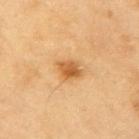Impression: The lesion was tiled from a total-body skin photograph and was not biopsied. Acquisition and patient details: A male subject, approximately 60 years of age. The lesion is on the arm. A 15 mm crop from a total-body photograph taken for skin-cancer surveillance. The lesion's longest dimension is about 3 mm.